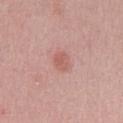Captured during whole-body skin photography for melanoma surveillance; the lesion was not biopsied. Automated image analysis of the tile measured an area of roughly 3 mm², an eccentricity of roughly 0.8, and two-axis asymmetry of about 0.35. And it measured a mean CIELAB color near L≈58 a*≈25 b*≈26, a lesion–skin lightness drop of about 8, and a normalized lesion–skin contrast near 6. The analysis additionally found a nevus-likeness score of about 25/100 and a detector confidence of about 100 out of 100 that the crop contains a lesion. Located on the chest. A male patient, aged 48 to 52. A region of skin cropped from a whole-body photographic capture, roughly 15 mm wide. The recorded lesion diameter is about 2.5 mm.